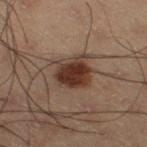<lesion>
<biopsy_status>not biopsied; imaged during a skin examination</biopsy_status>
<site>leg</site>
<patient>
  <sex>male</sex>
  <age_approx>55</age_approx>
</patient>
<lighting>cross-polarized</lighting>
<image>
  <source>total-body photography crop</source>
  <field_of_view_mm>15</field_of_view_mm>
</image>
<lesion_size>
  <long_diameter_mm_approx>4.5</long_diameter_mm_approx>
</lesion_size>
</lesion>